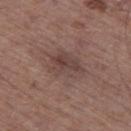Imaged during a routine full-body skin examination; the lesion was not biopsied and no histopathology is available. A region of skin cropped from a whole-body photographic capture, roughly 15 mm wide. Captured under white-light illumination. From the left thigh. Automated image analysis of the tile measured a footprint of about 10 mm², an eccentricity of roughly 0.75, and two-axis asymmetry of about 0.25. It also reported a lesion color around L≈42 a*≈17 b*≈20 in CIELAB, roughly 7 lightness units darker than nearby skin, and a normalized border contrast of about 6. The analysis additionally found a border-irregularity index near 3/10, a color-variation rating of about 4.5/10, and radial color variation of about 1.5. A male patient, roughly 65 years of age. The recorded lesion diameter is about 4.5 mm.